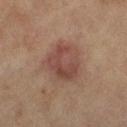follow-up: catalogued during a skin exam; not biopsied | automated metrics: an area of roughly 18 mm², an eccentricity of roughly 0.5, and a shape-asymmetry score of about 0.2 (0 = symmetric); a lesion color around L≈40 a*≈18 b*≈22 in CIELAB, a lesion–skin lightness drop of about 7, and a lesion-to-skin contrast of about 6.5 (normalized; higher = more distinct); a nevus-likeness score of about 95/100 and lesion-presence confidence of about 100/100 | site: the left thigh | lighting: cross-polarized illumination | image: ~15 mm crop, total-body skin-cancer survey | lesion size: about 5.5 mm | patient: female, aged 58–62.